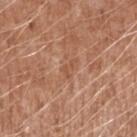The lesion was photographed on a routine skin check and not biopsied; there is no pathology result. The lesion is located on the right upper arm. The recorded lesion diameter is about 2.5 mm. Cropped from a whole-body photographic skin survey; the tile spans about 15 mm. A male subject, in their mid- to late 40s. Imaged with white-light lighting.